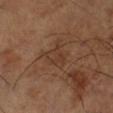Background: The subject is a male aged around 65. Longest diameter approximately 3 mm. The lesion is located on the right lower leg. This is a cross-polarized tile. A 15 mm close-up tile from a total-body photography series done for melanoma screening. The lesion-visualizer software estimated a lesion color around L≈37 a*≈18 b*≈28 in CIELAB and about 5 CIELAB-L* units darker than the surrounding skin. And it measured a border-irregularity rating of about 3.5/10 and radial color variation of about 1.